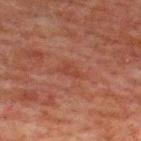subject=male, about 60 years old; diameter=~3 mm (longest diameter); anatomic site=the upper back; acquisition=total-body-photography crop, ~15 mm field of view.